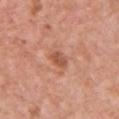notes=imaged on a skin check; not biopsied